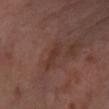The lesion was tiled from a total-body skin photograph and was not biopsied. The lesion is located on the left forearm. The subject is a female roughly 55 years of age. A lesion tile, about 15 mm wide, cut from a 3D total-body photograph.